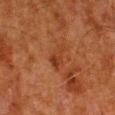The lesion was tiled from a total-body skin photograph and was not biopsied. The subject is a male aged around 80. The lesion is on the right lower leg. A roughly 15 mm field-of-view crop from a total-body skin photograph. The total-body-photography lesion software estimated an area of roughly 4.5 mm², an outline eccentricity of about 0.85 (0 = round, 1 = elongated), and a shape-asymmetry score of about 0.45 (0 = symmetric). And it measured a mean CIELAB color near L≈31 a*≈23 b*≈30, roughly 6 lightness units darker than nearby skin, and a normalized border contrast of about 6. Imaged with cross-polarized lighting. Longest diameter approximately 3.5 mm.